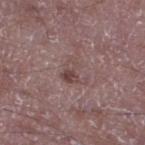{
  "biopsy_status": "not biopsied; imaged during a skin examination",
  "lighting": "white-light",
  "lesion_size": {
    "long_diameter_mm_approx": 3.5
  },
  "image": {
    "source": "total-body photography crop",
    "field_of_view_mm": 15
  },
  "site": "left lower leg",
  "patient": {
    "sex": "male",
    "age_approx": 50
  }
}